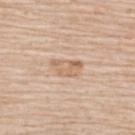notes = catalogued during a skin exam; not biopsied
size = ≈4 mm
lighting = white-light illumination
anatomic site = the upper back
acquisition = total-body-photography crop, ~15 mm field of view
subject = male, aged 78–82